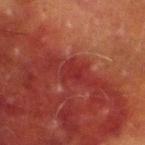Clinical impression:
Captured during whole-body skin photography for melanoma surveillance; the lesion was not biopsied.
Image and clinical context:
A male subject approximately 70 years of age. Automated tile analysis of the lesion measured two-axis asymmetry of about 0.3. It also reported a lesion–skin lightness drop of about 5. It also reported a color-variation rating of about 2/10. Captured under cross-polarized illumination. Located on the leg. A roughly 15 mm field-of-view crop from a total-body skin photograph.